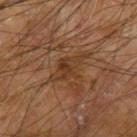Clinical impression: The lesion was photographed on a routine skin check and not biopsied; there is no pathology result. Clinical summary: A lesion tile, about 15 mm wide, cut from a 3D total-body photograph. On the left upper arm. Approximately 5 mm at its widest. The patient is a male approximately 65 years of age.